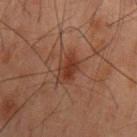Imaged during a routine full-body skin examination; the lesion was not biopsied and no histopathology is available. A male subject aged approximately 60. On the mid back. A close-up tile cropped from a whole-body skin photograph, about 15 mm across.